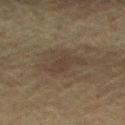Clinical impression:
Recorded during total-body skin imaging; not selected for excision or biopsy.
Background:
The patient is a male aged 63–67. The lesion is on the right upper arm. A roughly 15 mm field-of-view crop from a total-body skin photograph.